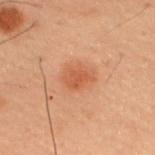Notes:
- workup: no biopsy performed (imaged during a skin exam)
- patient: male, approximately 55 years of age
- location: the upper back
- imaging modality: total-body-photography crop, ~15 mm field of view
- lesion diameter: about 4 mm
- TBP lesion metrics: an area of roughly 8 mm², an outline eccentricity of about 0.7 (0 = round, 1 = elongated), and a symmetry-axis asymmetry near 0.15; a lesion color around L≈46 a*≈23 b*≈32 in CIELAB, a lesion–skin lightness drop of about 7, and a normalized border contrast of about 6; border irregularity of about 1.5 on a 0–10 scale, a within-lesion color-variation index near 2.5/10, and radial color variation of about 0.5